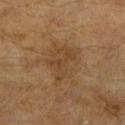  biopsy_status: not biopsied; imaged during a skin examination
  patient:
    sex: female
    age_approx: 60
  site: right forearm
  image:
    source: total-body photography crop
    field_of_view_mm: 15